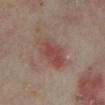| feature | finding |
|---|---|
| notes | total-body-photography surveillance lesion; no biopsy |